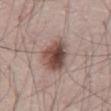workup = catalogued during a skin exam; not biopsied | location = the abdomen | image source = ~15 mm crop, total-body skin-cancer survey | lighting = white-light | subject = male, in their mid-60s | lesion size = about 4 mm | automated metrics = an average lesion color of about L≈48 a*≈18 b*≈22 (CIELAB) and a normalized border contrast of about 10.5; a nevus-likeness score of about 95/100 and a lesion-detection confidence of about 100/100.